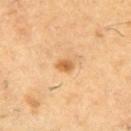The subject is a male roughly 60 years of age.
From the left thigh.
A lesion tile, about 15 mm wide, cut from a 3D total-body photograph.
The lesion's longest dimension is about 2 mm.
Captured under cross-polarized illumination.